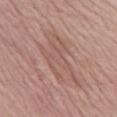Imaged during a routine full-body skin examination; the lesion was not biopsied and no histopathology is available. Located on the chest. A 15 mm close-up tile from a total-body photography series done for melanoma screening. Longest diameter approximately 8 mm. Captured under white-light illumination. The subject is a male approximately 85 years of age or older.